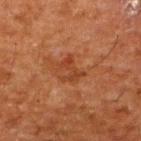biopsy status: imaged on a skin check; not biopsied | site: the upper back | size: ≈3 mm | imaging modality: ~15 mm crop, total-body skin-cancer survey | subject: male, roughly 60 years of age | lighting: cross-polarized illumination.